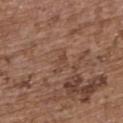Q: Was a biopsy performed?
A: imaged on a skin check; not biopsied
Q: What kind of image is this?
A: ~15 mm tile from a whole-body skin photo
Q: Lesion location?
A: the upper back
Q: Patient demographics?
A: female, aged approximately 65
Q: What is the lesion's diameter?
A: about 3 mm
Q: Illumination type?
A: white-light illumination